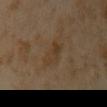follow-up: no biopsy performed (imaged during a skin exam); patient: female, approximately 35 years of age; lesion diameter: ≈4 mm; anatomic site: the front of the torso; image source: ~15 mm tile from a whole-body skin photo.